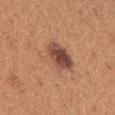No biopsy was performed on this lesion — it was imaged during a full skin examination and was not determined to be concerning. Imaged with white-light lighting. Measured at roughly 5 mm in maximum diameter. A region of skin cropped from a whole-body photographic capture, roughly 15 mm wide. The subject is a female about 45 years old. Located on the arm.